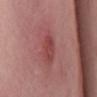Clinical impression: Captured during whole-body skin photography for melanoma surveillance; the lesion was not biopsied. Background: Automated image analysis of the tile measured a footprint of about 6.5 mm² and a shape eccentricity near 0.65. And it measured a mean CIELAB color near L≈45 a*≈29 b*≈23, roughly 8 lightness units darker than nearby skin, and a normalized border contrast of about 6.5. The analysis additionally found lesion-presence confidence of about 100/100. This is a white-light tile. From the mid back. A female subject in their mid- to late 60s. The lesion's longest dimension is about 3.5 mm. A region of skin cropped from a whole-body photographic capture, roughly 15 mm wide.